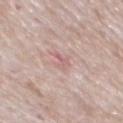Q: Automated lesion metrics?
A: an average lesion color of about L≈62 a*≈22 b*≈20 (CIELAB) and a lesion–skin lightness drop of about 7
Q: How was this image acquired?
A: 15 mm crop, total-body photography
Q: Where on the body is the lesion?
A: the mid back
Q: How was the tile lit?
A: white-light illumination
Q: Lesion size?
A: ≈2.5 mm
Q: Patient demographics?
A: male, aged around 75